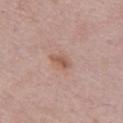follow-up: catalogued during a skin exam; not biopsied | image source: ~15 mm crop, total-body skin-cancer survey | subject: female, aged 58–62 | body site: the chest.